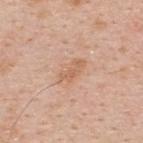workup = no biopsy performed (imaged during a skin exam)
automated metrics = an area of roughly 6.5 mm², a shape eccentricity near 0.85, and two-axis asymmetry of about 0.2; an average lesion color of about L≈63 a*≈20 b*≈33 (CIELAB), roughly 7 lightness units darker than nearby skin, and a normalized lesion–skin contrast near 5.5; a border-irregularity index near 2.5/10, internal color variation of about 2.5 on a 0–10 scale, and peripheral color asymmetry of about 1; an automated nevus-likeness rating near 0 out of 100 and a lesion-detection confidence of about 100/100
site = the upper back
acquisition = ~15 mm tile from a whole-body skin photo
subject = male, about 35 years old